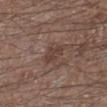Part of a total-body skin-imaging series; this lesion was reviewed on a skin check and was not flagged for biopsy. A male subject, aged around 65. On the left lower leg. Imaged with white-light lighting. The lesion-visualizer software estimated a lesion area of about 6 mm², an eccentricity of roughly 0.7, and a symmetry-axis asymmetry near 0.25. It also reported a normalized lesion–skin contrast near 6. And it measured internal color variation of about 2 on a 0–10 scale and radial color variation of about 0.5. It also reported a lesion-detection confidence of about 100/100. A 15 mm close-up extracted from a 3D total-body photography capture.